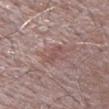The lesion was tiled from a total-body skin photograph and was not biopsied. The lesion is on the left upper arm. An algorithmic analysis of the crop reported a lesion–skin lightness drop of about 7 and a lesion-to-skin contrast of about 5 (normalized; higher = more distinct). It also reported a border-irregularity index near 4.5/10, a within-lesion color-variation index near 0/10, and radial color variation of about 0. It also reported an automated nevus-likeness rating near 0 out of 100. Imaged with white-light lighting. A 15 mm close-up tile from a total-body photography series done for melanoma screening. A male patient, in their mid- to late 60s. The recorded lesion diameter is about 3 mm.